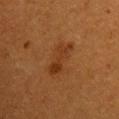Part of a total-body skin-imaging series; this lesion was reviewed on a skin check and was not flagged for biopsy.
A female patient aged 53 to 57.
This image is a 15 mm lesion crop taken from a total-body photograph.
The lesion's longest dimension is about 5 mm.
On the front of the torso.